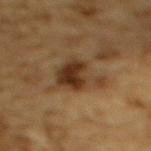Captured during whole-body skin photography for melanoma surveillance; the lesion was not biopsied. Imaged with cross-polarized lighting. A male subject in their mid-80s or older. From the upper back. Cropped from a whole-body photographic skin survey; the tile spans about 15 mm. The lesion's longest dimension is about 7 mm.